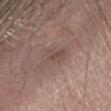Q: Is there a histopathology result?
A: total-body-photography surveillance lesion; no biopsy
Q: Lesion size?
A: ~3.5 mm (longest diameter)
Q: Lesion location?
A: the head or neck
Q: Who is the patient?
A: female, in their mid- to late 30s
Q: Automated lesion metrics?
A: a mean CIELAB color near L≈47 a*≈17 b*≈22 and a normalized lesion–skin contrast near 5.5; a nevus-likeness score of about 0/100
Q: What lighting was used for the tile?
A: white-light illumination
Q: What kind of image is this?
A: total-body-photography crop, ~15 mm field of view